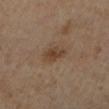Impression:
This lesion was catalogued during total-body skin photography and was not selected for biopsy.
Context:
From the left lower leg. A male subject aged approximately 65. A lesion tile, about 15 mm wide, cut from a 3D total-body photograph.